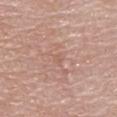– patient: female, aged around 65
– lesion size: ~1 mm (longest diameter)
– anatomic site: the upper back
– image: ~15 mm crop, total-body skin-cancer survey